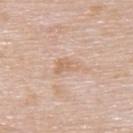– notes: no biopsy performed (imaged during a skin exam)
– patient: female, aged 58 to 62
– image source: total-body-photography crop, ~15 mm field of view
– body site: the upper back
– tile lighting: white-light
– automated lesion analysis: a lesion color around L≈65 a*≈18 b*≈32 in CIELAB, about 7 CIELAB-L* units darker than the surrounding skin, and a normalized lesion–skin contrast near 6